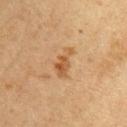Imaged during a routine full-body skin examination; the lesion was not biopsied and no histopathology is available.